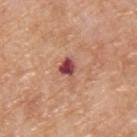biopsy status = total-body-photography surveillance lesion; no biopsy | illumination = white-light | subject = male, in their mid- to late 50s | TBP lesion metrics = an average lesion color of about L≈48 a*≈29 b*≈24 (CIELAB), about 16 CIELAB-L* units darker than the surrounding skin, and a normalized lesion–skin contrast near 12; a border-irregularity rating of about 3/10 and a peripheral color-asymmetry measure near 1.5 | image source = ~15 mm tile from a whole-body skin photo | size = ≈2.5 mm | location = the upper back.